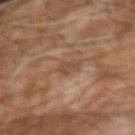Notes:
* notes: total-body-photography surveillance lesion; no biopsy
* automated lesion analysis: a footprint of about 2.5 mm², a shape eccentricity near 0.85, and a shape-asymmetry score of about 0.45 (0 = symmetric); a lesion color around L≈43 a*≈17 b*≈28 in CIELAB and a normalized border contrast of about 6; a border-irregularity index near 4.5/10, a within-lesion color-variation index near 0.5/10, and a peripheral color-asymmetry measure near 0
* subject: male, in their mid-50s
* lesion size: ≈2.5 mm
* lighting: cross-polarized illumination
* image: ~15 mm crop, total-body skin-cancer survey
* site: the right forearm